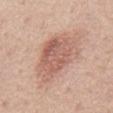Q: Was a biopsy performed?
A: catalogued during a skin exam; not biopsied
Q: What lighting was used for the tile?
A: white-light illumination
Q: How large is the lesion?
A: ≈7 mm
Q: What is the imaging modality?
A: ~15 mm tile from a whole-body skin photo
Q: Who is the patient?
A: male, aged around 50
Q: What is the anatomic site?
A: the chest
Q: What did automated image analysis measure?
A: a lesion area of about 23 mm²